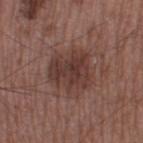Captured during whole-body skin photography for melanoma surveillance; the lesion was not biopsied. The recorded lesion diameter is about 5 mm. The total-body-photography lesion software estimated a footprint of about 15 mm² and an outline eccentricity of about 0.4 (0 = round, 1 = elongated). The analysis additionally found a mean CIELAB color near L≈36 a*≈19 b*≈22, about 9 CIELAB-L* units darker than the surrounding skin, and a normalized border contrast of about 7.5. The software also gave a nevus-likeness score of about 15/100. This is a white-light tile. A roughly 15 mm field-of-view crop from a total-body skin photograph. The lesion is on the leg. A male patient aged 48 to 52.